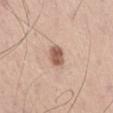A male patient in their mid-50s.
A close-up tile cropped from a whole-body skin photograph, about 15 mm across.
The lesion is on the abdomen.